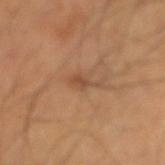<case>
<biopsy_status>not biopsied; imaged during a skin examination</biopsy_status>
<lighting>cross-polarized</lighting>
<image>
  <source>total-body photography crop</source>
  <field_of_view_mm>15</field_of_view_mm>
</image>
<patient>
  <sex>male</sex>
  <age_approx>50</age_approx>
</patient>
<site>right forearm</site>
<lesion_size>
  <long_diameter_mm_approx>3.5</long_diameter_mm_approx>
</lesion_size>
</case>